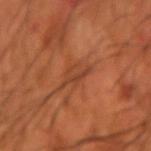This lesion was catalogued during total-body skin photography and was not selected for biopsy. Approximately 4 mm at its widest. An algorithmic analysis of the crop reported a shape eccentricity near 0.9 and a shape-asymmetry score of about 0.5 (0 = symmetric). And it measured a border-irregularity index near 9/10 and peripheral color asymmetry of about 0. Located on the left forearm. A 15 mm crop from a total-body photograph taken for skin-cancer surveillance. A male patient, aged 68 to 72. Imaged with cross-polarized lighting.